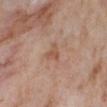| field | value |
|---|---|
| workup | total-body-photography surveillance lesion; no biopsy |